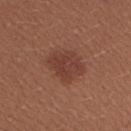Background:
The patient is a female aged around 25. Imaged with white-light lighting. This image is a 15 mm lesion crop taken from a total-body photograph. The lesion is located on the arm.